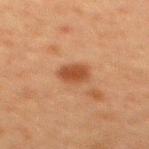Notes:
- follow-up — catalogued during a skin exam; not biopsied
- subject — male, aged approximately 60
- body site — the mid back
- lesion diameter — ≈3.5 mm
- lighting — cross-polarized
- image source — total-body-photography crop, ~15 mm field of view